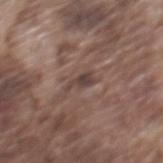Captured during whole-body skin photography for melanoma surveillance; the lesion was not biopsied.
The recorded lesion diameter is about 2.5 mm.
Imaged with white-light lighting.
A male subject approximately 75 years of age.
The lesion is on the mid back.
A 15 mm close-up extracted from a 3D total-body photography capture.